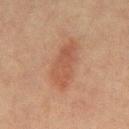| key | value |
|---|---|
| body site | the chest |
| automated metrics | about 7 CIELAB-L* units darker than the surrounding skin and a lesion-to-skin contrast of about 6 (normalized; higher = more distinct) |
| subject | male, aged 63 to 67 |
| tile lighting | cross-polarized illumination |
| image | ~15 mm tile from a whole-body skin photo |
| size | about 6.5 mm |